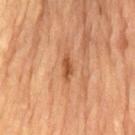This lesion was catalogued during total-body skin photography and was not selected for biopsy.
A close-up tile cropped from a whole-body skin photograph, about 15 mm across.
On the back.
The patient is a male about 85 years old or older.